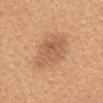Notes:
- workup · imaged on a skin check; not biopsied
- image source · 15 mm crop, total-body photography
- anatomic site · the head or neck
- TBP lesion metrics · a lesion color around L≈60 a*≈22 b*≈35 in CIELAB and a lesion–skin lightness drop of about 9; border irregularity of about 2 on a 0–10 scale, internal color variation of about 4 on a 0–10 scale, and peripheral color asymmetry of about 1.5; a classifier nevus-likeness of about 50/100 and a lesion-detection confidence of about 100/100
- tile lighting · white-light illumination
- lesion size · about 5.5 mm
- patient · female, in their mid- to late 20s